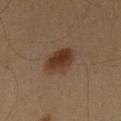notes = no biopsy performed (imaged during a skin exam) | patient = male, about 60 years old | image = ~15 mm tile from a whole-body skin photo | automated lesion analysis = a lesion area of about 9.5 mm², an eccentricity of roughly 0.7, and a symmetry-axis asymmetry near 0.15; a border-irregularity rating of about 1.5/10, a within-lesion color-variation index near 4/10, and radial color variation of about 1.5; a classifier nevus-likeness of about 100/100 | tile lighting = cross-polarized illumination | site = the chest | lesion diameter = ≈4 mm.